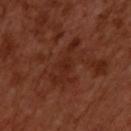follow-up: total-body-photography surveillance lesion; no biopsy | subject: male, in their 50s | TBP lesion metrics: a lesion area of about 8.5 mm², a shape eccentricity near 0.95, and a shape-asymmetry score of about 0.55 (0 = symmetric); an average lesion color of about L≈26 a*≈24 b*≈27 (CIELAB), roughly 5 lightness units darker than nearby skin, and a lesion-to-skin contrast of about 5.5 (normalized; higher = more distinct); a border-irregularity index near 9/10, a color-variation rating of about 1.5/10, and peripheral color asymmetry of about 0.5; an automated nevus-likeness rating near 0 out of 100 and a lesion-detection confidence of about 80/100 | image source: ~15 mm crop, total-body skin-cancer survey | lesion size: about 5.5 mm | body site: the upper back | illumination: cross-polarized illumination.